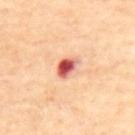Longest diameter approximately 3 mm. A 15 mm crop from a total-body photograph taken for skin-cancer surveillance. A male patient roughly 70 years of age. The lesion is located on the back. The tile uses cross-polarized illumination.